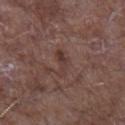Impression: Recorded during total-body skin imaging; not selected for excision or biopsy. Image and clinical context: The recorded lesion diameter is about 4 mm. The lesion is on the left forearm. A region of skin cropped from a whole-body photographic capture, roughly 15 mm wide. This is a white-light tile. The subject is a male approximately 65 years of age.